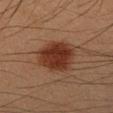follow-up: catalogued during a skin exam; not biopsied | site: the left forearm | acquisition: 15 mm crop, total-body photography | patient: male, approximately 35 years of age.